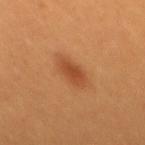{"image": {"source": "total-body photography crop", "field_of_view_mm": 15}, "lesion_size": {"long_diameter_mm_approx": 4.0}, "site": "mid back", "automated_metrics": {"cielab_L": 44, "cielab_a": 24, "cielab_b": 37, "vs_skin_darker_L": 9.0}, "patient": {"sex": "female", "age_approx": 30}}